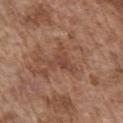Clinical impression:
This lesion was catalogued during total-body skin photography and was not selected for biopsy.
Acquisition and patient details:
The recorded lesion diameter is about 4 mm. The lesion is located on the chest. A male subject, aged approximately 75. The tile uses white-light illumination. A region of skin cropped from a whole-body photographic capture, roughly 15 mm wide.